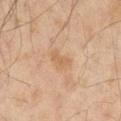Q: Is there a histopathology result?
A: total-body-photography surveillance lesion; no biopsy
Q: Patient demographics?
A: male, in their 60s
Q: What lighting was used for the tile?
A: cross-polarized
Q: Lesion location?
A: the right thigh
Q: What is the imaging modality?
A: total-body-photography crop, ~15 mm field of view
Q: Lesion size?
A: ~3 mm (longest diameter)